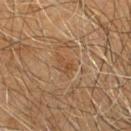  biopsy_status: not biopsied; imaged during a skin examination
  site: chest
  lighting: cross-polarized
  patient:
    sex: male
    age_approx: 60
  automated_metrics:
    border_irregularity_0_10: 4.5
    peripheral_color_asymmetry: 0.5
  image:
    source: total-body photography crop
    field_of_view_mm: 15
  lesion_size:
    long_diameter_mm_approx: 2.5